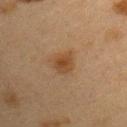workup — catalogued during a skin exam; not biopsied
patient — female, aged around 40
image — ~15 mm crop, total-body skin-cancer survey
body site — the left upper arm